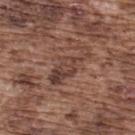{
  "biopsy_status": "not biopsied; imaged during a skin examination",
  "automated_metrics": {
    "area_mm2_approx": 9.0,
    "eccentricity": 0.95,
    "shape_asymmetry": 0.5,
    "cielab_L": 41,
    "cielab_a": 19,
    "cielab_b": 23,
    "vs_skin_darker_L": 8.0,
    "vs_skin_contrast_norm": 7.0,
    "nevus_likeness_0_100": 0,
    "lesion_detection_confidence_0_100": 55
  },
  "lighting": "white-light",
  "patient": {
    "sex": "male",
    "age_approx": 75
  },
  "site": "upper back",
  "image": {
    "source": "total-body photography crop",
    "field_of_view_mm": 15
  },
  "lesion_size": {
    "long_diameter_mm_approx": 6.0
  }
}